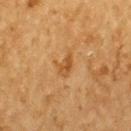- workup · no biopsy performed (imaged during a skin exam)
- acquisition · ~15 mm crop, total-body skin-cancer survey
- location · the upper back
- size · ≈3 mm
- patient · male, in their mid- to late 80s or older
- illumination · cross-polarized
- automated metrics · an eccentricity of roughly 0.8 and two-axis asymmetry of about 0.35; a mean CIELAB color near L≈46 a*≈20 b*≈38, a lesion–skin lightness drop of about 8, and a normalized border contrast of about 6.5; border irregularity of about 3.5 on a 0–10 scale, a color-variation rating of about 2/10, and a peripheral color-asymmetry measure near 0.5; an automated nevus-likeness rating near 0 out of 100